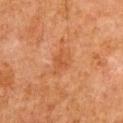The lesion was photographed on a routine skin check and not biopsied; there is no pathology result.
A male subject aged 78 to 82.
Imaged with cross-polarized lighting.
A region of skin cropped from a whole-body photographic capture, roughly 15 mm wide.
From the front of the torso.
Automated tile analysis of the lesion measured a mean CIELAB color near L≈42 a*≈23 b*≈33, about 5 CIELAB-L* units darker than the surrounding skin, and a normalized border contrast of about 5. It also reported a border-irregularity index near 4/10, a color-variation rating of about 2/10, and a peripheral color-asymmetry measure near 0.5. The software also gave lesion-presence confidence of about 100/100.
Approximately 3 mm at its widest.